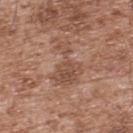Findings:
• biopsy status: total-body-photography surveillance lesion; no biopsy
• subject: male, in their mid- to late 50s
• imaging modality: 15 mm crop, total-body photography
• lesion diameter: about 6 mm
• anatomic site: the upper back